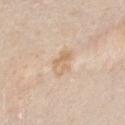Q: Was a biopsy performed?
A: catalogued during a skin exam; not biopsied
Q: What is the lesion's diameter?
A: about 3.5 mm
Q: Lesion location?
A: the chest
Q: What are the patient's age and sex?
A: female, about 20 years old
Q: What is the imaging modality?
A: total-body-photography crop, ~15 mm field of view